A 15 mm crop from a total-body photograph taken for skin-cancer surveillance · located on the arm · a male patient, in their 70s · longest diameter approximately 4.5 mm: 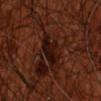Q: What did pathology find?
A: a seborrheic keratosis